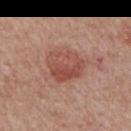Assessment: This lesion was catalogued during total-body skin photography and was not selected for biopsy.